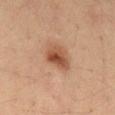biopsy_status: not biopsied; imaged during a skin examination
lighting: cross-polarized
patient:
  sex: male
  age_approx: 35
site: lower back
lesion_size:
  long_diameter_mm_approx: 3.5
image:
  source: total-body photography crop
  field_of_view_mm: 15